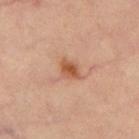notes: no biopsy performed (imaged during a skin exam)
automated lesion analysis: a border-irregularity index near 2/10, a color-variation rating of about 2.5/10, and a peripheral color-asymmetry measure near 0.5; an automated nevus-likeness rating near 90 out of 100 and a lesion-detection confidence of about 100/100
image: ~15 mm crop, total-body skin-cancer survey
site: the left thigh
subject: female, aged around 65
illumination: cross-polarized